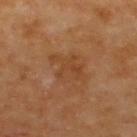Impression: The lesion was photographed on a routine skin check and not biopsied; there is no pathology result. Background: From the upper back. Imaged with cross-polarized lighting. The lesion's longest dimension is about 4 mm. A 15 mm close-up extracted from a 3D total-body photography capture. The subject is approximately 65 years of age.